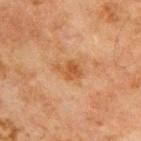{"biopsy_status": "not biopsied; imaged during a skin examination", "image": {"source": "total-body photography crop", "field_of_view_mm": 15}, "lesion_size": {"long_diameter_mm_approx": 3.0}, "patient": {"sex": "male", "age_approx": 65}, "automated_metrics": {"cielab_L": 43, "cielab_a": 20, "cielab_b": 34, "vs_skin_contrast_norm": 7.5, "border_irregularity_0_10": 4.0, "color_variation_0_10": 2.0}, "site": "chest", "lighting": "cross-polarized"}